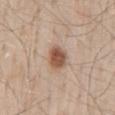The lesion was tiled from a total-body skin photograph and was not biopsied. From the mid back. This image is a 15 mm lesion crop taken from a total-body photograph. A male patient, aged approximately 60. About 3 mm across.